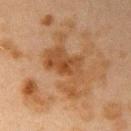Q: Was this lesion biopsied?
A: imaged on a skin check; not biopsied
Q: What are the patient's age and sex?
A: female, aged 38 to 42
Q: What kind of image is this?
A: ~15 mm tile from a whole-body skin photo
Q: What did automated image analysis measure?
A: a lesion–skin lightness drop of about 8; an automated nevus-likeness rating near 0 out of 100
Q: What is the anatomic site?
A: the right upper arm
Q: How large is the lesion?
A: ≈7.5 mm